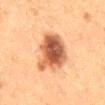Recorded during total-body skin imaging; not selected for excision or biopsy. This is a cross-polarized tile. An algorithmic analysis of the crop reported a nevus-likeness score of about 95/100 and lesion-presence confidence of about 100/100. Longest diameter approximately 6.5 mm. The lesion is on the mid back. A roughly 15 mm field-of-view crop from a total-body skin photograph. A male subject aged around 50.